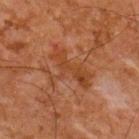Assessment: Imaged during a routine full-body skin examination; the lesion was not biopsied and no histopathology is available. Clinical summary: On the upper back. The total-body-photography lesion software estimated a detector confidence of about 100 out of 100 that the crop contains a lesion. A male patient aged approximately 65. A lesion tile, about 15 mm wide, cut from a 3D total-body photograph. Imaged with cross-polarized lighting.